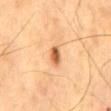image source = ~15 mm tile from a whole-body skin photo | subject = male, approximately 60 years of age | location = the mid back | image-analysis metrics = an area of roughly 3.5 mm²; an average lesion color of about L≈62 a*≈26 b*≈41 (CIELAB), roughly 16 lightness units darker than nearby skin, and a normalized lesion–skin contrast near 9.5; a border-irregularity rating of about 2/10, internal color variation of about 4 on a 0–10 scale, and a peripheral color-asymmetry measure near 1.5.